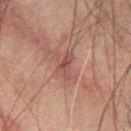The lesion was photographed on a routine skin check and not biopsied; there is no pathology result.
The lesion-visualizer software estimated an average lesion color of about L≈36 a*≈19 b*≈18 (CIELAB), roughly 7 lightness units darker than nearby skin, and a normalized border contrast of about 6.5. The software also gave a color-variation rating of about 2.5/10.
Imaged with cross-polarized lighting.
Located on the leg.
About 3.5 mm across.
A male patient, aged around 60.
Cropped from a total-body skin-imaging series; the visible field is about 15 mm.